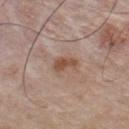The lesion was photographed on a routine skin check and not biopsied; there is no pathology result. The recorded lesion diameter is about 3 mm. A roughly 15 mm field-of-view crop from a total-body skin photograph. Located on the chest. A male patient, aged 53–57. Imaged with white-light lighting. The lesion-visualizer software estimated a shape eccentricity near 0.85. The analysis additionally found a mean CIELAB color near L≈50 a*≈19 b*≈29 and a normalized border contrast of about 8. The software also gave internal color variation of about 2.5 on a 0–10 scale and peripheral color asymmetry of about 0.5.